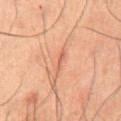notes: catalogued during a skin exam; not biopsied
location: the abdomen
image-analysis metrics: a mean CIELAB color near L≈47 a*≈21 b*≈27, a lesion–skin lightness drop of about 6, and a normalized border contrast of about 5; lesion-presence confidence of about 80/100
illumination: cross-polarized
image: total-body-photography crop, ~15 mm field of view
diameter: ≈2.5 mm
subject: male, roughly 50 years of age